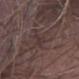imaging modality = 15 mm crop, total-body photography; anatomic site = the leg; patient = male, approximately 50 years of age; lesion diameter = ≈3 mm; illumination = white-light illumination; automated lesion analysis = an automated nevus-likeness rating near 0 out of 100 and a lesion-detection confidence of about 55/100.